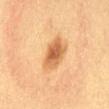Part of a total-body skin-imaging series; this lesion was reviewed on a skin check and was not flagged for biopsy. A roughly 15 mm field-of-view crop from a total-body skin photograph. A female patient aged around 30. Approximately 4.5 mm at its widest. From the abdomen. Automated tile analysis of the lesion measured a normalized lesion–skin contrast near 8. And it measured a nevus-likeness score of about 95/100 and a lesion-detection confidence of about 100/100.